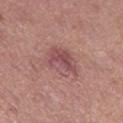Longest diameter approximately 4.5 mm.
Imaged with white-light lighting.
The lesion is located on the left thigh.
A female subject aged approximately 40.
A 15 mm close-up extracted from a 3D total-body photography capture.